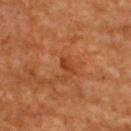notes: total-body-photography surveillance lesion; no biopsy | subject: female, about 65 years old | site: the upper back | acquisition: 15 mm crop, total-body photography | illumination: cross-polarized | diameter: ~2 mm (longest diameter) | automated metrics: a border-irregularity index near 2/10, internal color variation of about 0 on a 0–10 scale, and peripheral color asymmetry of about 0; a classifier nevus-likeness of about 0/100 and a detector confidence of about 100 out of 100 that the crop contains a lesion.